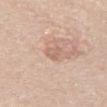Part of a total-body skin-imaging series; this lesion was reviewed on a skin check and was not flagged for biopsy.
A roughly 15 mm field-of-view crop from a total-body skin photograph.
The lesion is on the front of the torso.
A male subject, about 75 years old.